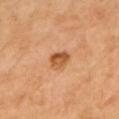follow-up=no biopsy performed (imaged during a skin exam)
subject=female, aged approximately 70
automated lesion analysis=an area of roughly 5 mm², an eccentricity of roughly 0.65, and two-axis asymmetry of about 0.2; a border-irregularity rating of about 2/10, a color-variation rating of about 5.5/10, and radial color variation of about 2
lesion size=~3 mm (longest diameter)
imaging modality=~15 mm crop, total-body skin-cancer survey
location=the chest